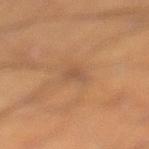Q: Was a biopsy performed?
A: no biopsy performed (imaged during a skin exam)
Q: What is the anatomic site?
A: the leg
Q: What is the imaging modality?
A: ~15 mm tile from a whole-body skin photo
Q: What are the patient's age and sex?
A: male, about 55 years old